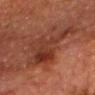Impression: No biopsy was performed on this lesion — it was imaged during a full skin examination and was not determined to be concerning. Context: A close-up tile cropped from a whole-body skin photograph, about 15 mm across. The tile uses cross-polarized illumination. The lesion is located on the head or neck. The subject is a male aged 78 to 82. The recorded lesion diameter is about 8 mm.